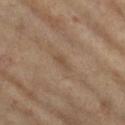| feature | finding |
|---|---|
| biopsy status | total-body-photography surveillance lesion; no biopsy |
| body site | the left lower leg |
| subject | female, aged 73 to 77 |
| acquisition | 15 mm crop, total-body photography |